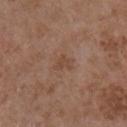notes: imaged on a skin check; not biopsied
TBP lesion metrics: a color-variation rating of about 1/10; an automated nevus-likeness rating near 0 out of 100 and a lesion-detection confidence of about 100/100
image source: total-body-photography crop, ~15 mm field of view
size: ≈2.5 mm
patient: male, in their mid-50s
body site: the right upper arm
lighting: white-light illumination